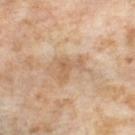{
  "biopsy_status": "not biopsied; imaged during a skin examination",
  "site": "right thigh",
  "patient": {
    "sex": "female",
    "age_approx": 55
  },
  "automated_metrics": {
    "cielab_L": 62,
    "cielab_a": 18,
    "cielab_b": 34,
    "border_irregularity_0_10": 7.5,
    "nevus_likeness_0_100": 0,
    "lesion_detection_confidence_0_100": 100
  },
  "image": {
    "source": "total-body photography crop",
    "field_of_view_mm": 15
  }
}